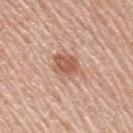notes=no biopsy performed (imaged during a skin exam) | lesion diameter=about 4.5 mm | TBP lesion metrics=an area of roughly 8 mm²; a border-irregularity index near 3/10 and a within-lesion color-variation index near 2.5/10; a nevus-likeness score of about 80/100 and a lesion-detection confidence of about 100/100 | acquisition=15 mm crop, total-body photography | body site=the right upper arm | lighting=white-light illumination | patient=female, aged 63–67.